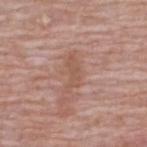Part of a total-body skin-imaging series; this lesion was reviewed on a skin check and was not flagged for biopsy. The lesion is on the back. A roughly 15 mm field-of-view crop from a total-body skin photograph. Automated tile analysis of the lesion measured a shape eccentricity near 0.9 and two-axis asymmetry of about 0.55. It also reported an average lesion color of about L≈54 a*≈20 b*≈28 (CIELAB), roughly 7 lightness units darker than nearby skin, and a lesion-to-skin contrast of about 5.5 (normalized; higher = more distinct). The analysis additionally found border irregularity of about 6 on a 0–10 scale, a within-lesion color-variation index near 1/10, and a peripheral color-asymmetry measure near 0. And it measured a classifier nevus-likeness of about 0/100. The subject is a male aged approximately 65. Measured at roughly 4 mm in maximum diameter. The tile uses white-light illumination.